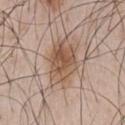Context:
An algorithmic analysis of the crop reported an eccentricity of roughly 0.8. The analysis additionally found border irregularity of about 3 on a 0–10 scale and internal color variation of about 6.5 on a 0–10 scale. The software also gave an automated nevus-likeness rating near 90 out of 100 and lesion-presence confidence of about 100/100. A close-up tile cropped from a whole-body skin photograph, about 15 mm across. A male patient aged around 50. Longest diameter approximately 5.5 mm. The lesion is located on the front of the torso.